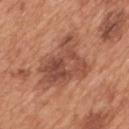Clinical impression:
Recorded during total-body skin imaging; not selected for excision or biopsy.
Acquisition and patient details:
The total-body-photography lesion software estimated a lesion–skin lightness drop of about 11. The recorded lesion diameter is about 6.5 mm. A male subject aged around 65. This is a white-light tile. Located on the mid back. Cropped from a whole-body photographic skin survey; the tile spans about 15 mm.